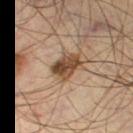notes: imaged on a skin check; not biopsied
diameter: about 3.5 mm
acquisition: ~15 mm tile from a whole-body skin photo
subject: male, aged 53 to 57
lighting: cross-polarized illumination
location: the left thigh